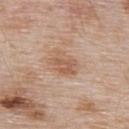{
  "biopsy_status": "not biopsied; imaged during a skin examination",
  "lesion_size": {
    "long_diameter_mm_approx": 3.5
  },
  "site": "upper back",
  "automated_metrics": {
    "border_irregularity_0_10": 2.0,
    "color_variation_0_10": 3.0,
    "peripheral_color_asymmetry": 1.0,
    "lesion_detection_confidence_0_100": 100
  },
  "image": {
    "source": "total-body photography crop",
    "field_of_view_mm": 15
  },
  "lighting": "white-light",
  "patient": {
    "sex": "male",
    "age_approx": 55
  }
}